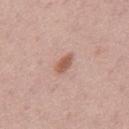Impression: No biopsy was performed on this lesion — it was imaged during a full skin examination and was not determined to be concerning. Acquisition and patient details: From the mid back. This image is a 15 mm lesion crop taken from a total-body photograph. The recorded lesion diameter is about 3 mm. The total-body-photography lesion software estimated a footprint of about 4 mm², an eccentricity of roughly 0.85, and a shape-asymmetry score of about 0.2 (0 = symmetric). It also reported a lesion color around L≈57 a*≈22 b*≈28 in CIELAB, roughly 11 lightness units darker than nearby skin, and a lesion-to-skin contrast of about 7.5 (normalized; higher = more distinct). And it measured a border-irregularity rating of about 2/10, a within-lesion color-variation index near 2/10, and peripheral color asymmetry of about 0.5. The analysis additionally found a classifier nevus-likeness of about 90/100 and lesion-presence confidence of about 100/100. A male subject, about 45 years old. Imaged with white-light lighting.